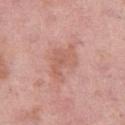Assessment: No biopsy was performed on this lesion — it was imaged during a full skin examination and was not determined to be concerning. Background: This is a white-light tile. A roughly 15 mm field-of-view crop from a total-body skin photograph. Measured at roughly 4.5 mm in maximum diameter. On the left thigh. A female patient, in their 50s. Automated tile analysis of the lesion measured a lesion color around L≈60 a*≈24 b*≈28 in CIELAB and roughly 7 lightness units darker than nearby skin. And it measured a border-irregularity index near 5/10, a color-variation rating of about 2/10, and peripheral color asymmetry of about 0.5.